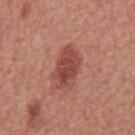| feature | finding |
|---|---|
| follow-up | total-body-photography surveillance lesion; no biopsy |
| TBP lesion metrics | a lesion color around L≈46 a*≈28 b*≈26 in CIELAB and a lesion-to-skin contrast of about 8.5 (normalized; higher = more distinct); a nevus-likeness score of about 90/100 and lesion-presence confidence of about 100/100 |
| illumination | white-light illumination |
| imaging modality | ~15 mm tile from a whole-body skin photo |
| anatomic site | the mid back |
| diameter | ~5 mm (longest diameter) |
| patient | male, in their mid-60s |